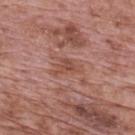Assessment: Imaged during a routine full-body skin examination; the lesion was not biopsied and no histopathology is available. Clinical summary: The lesion is located on the back. The total-body-photography lesion software estimated an area of roughly 5.5 mm², a shape eccentricity near 0.85, and two-axis asymmetry of about 0.45. Cropped from a total-body skin-imaging series; the visible field is about 15 mm. The patient is a male in their 70s. This is a white-light tile.